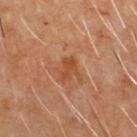No biopsy was performed on this lesion — it was imaged during a full skin examination and was not determined to be concerning.
A roughly 15 mm field-of-view crop from a total-body skin photograph.
Captured under cross-polarized illumination.
A male subject aged around 60.
The lesion's longest dimension is about 3 mm.
On the front of the torso.